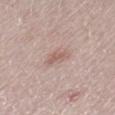Acquisition and patient details: A male subject, in their mid-70s. The lesion-visualizer software estimated an area of roughly 4 mm² and a shape eccentricity near 0.75. The analysis additionally found a lesion color around L≈59 a*≈19 b*≈23 in CIELAB, a lesion–skin lightness drop of about 8, and a lesion-to-skin contrast of about 6 (normalized; higher = more distinct). And it measured an automated nevus-likeness rating near 35 out of 100 and a detector confidence of about 100 out of 100 that the crop contains a lesion. The lesion is on the left thigh. A 15 mm close-up extracted from a 3D total-body photography capture.